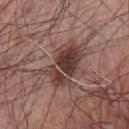workup: imaged on a skin check; not biopsied
tile lighting: white-light illumination
patient: male, about 65 years old
lesion size: about 5.5 mm
imaging modality: ~15 mm crop, total-body skin-cancer survey
anatomic site: the chest